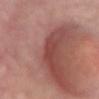Cropped from a whole-body photographic skin survey; the tile spans about 15 mm. Located on the upper back. A female subject roughly 75 years of age.